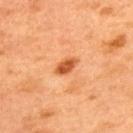The lesion was photographed on a routine skin check and not biopsied; there is no pathology result. The lesion is located on the upper back. A 15 mm crop from a total-body photograph taken for skin-cancer surveillance. The recorded lesion diameter is about 3 mm. Captured under cross-polarized illumination. Automated tile analysis of the lesion measured a nevus-likeness score of about 85/100 and a detector confidence of about 100 out of 100 that the crop contains a lesion. A male patient, aged 48 to 52.